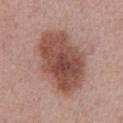Context:
From the chest. Cropped from a whole-body photographic skin survey; the tile spans about 15 mm. A male patient, in their 60s. The total-body-photography lesion software estimated border irregularity of about 2 on a 0–10 scale and peripheral color asymmetry of about 2. The software also gave lesion-presence confidence of about 100/100.